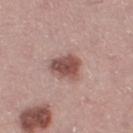The lesion was photographed on a routine skin check and not biopsied; there is no pathology result.